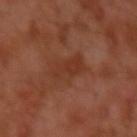Recorded during total-body skin imaging; not selected for excision or biopsy. Imaged with cross-polarized lighting. The lesion is located on the left upper arm. An algorithmic analysis of the crop reported an outline eccentricity of about 0.9 (0 = round, 1 = elongated) and a shape-asymmetry score of about 0.25 (0 = symmetric). The analysis additionally found an average lesion color of about L≈34 a*≈24 b*≈30 (CIELAB) and a normalized lesion–skin contrast near 5.5. It also reported an automated nevus-likeness rating near 0 out of 100 and a detector confidence of about 100 out of 100 that the crop contains a lesion. The subject is a male approximately 30 years of age. A 15 mm close-up tile from a total-body photography series done for melanoma screening.